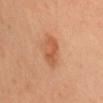No biopsy was performed on this lesion — it was imaged during a full skin examination and was not determined to be concerning. A female patient aged around 40. Located on the chest. The recorded lesion diameter is about 4 mm. A 15 mm crop from a total-body photograph taken for skin-cancer surveillance.